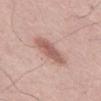site = the abdomen
imaging modality = total-body-photography crop, ~15 mm field of view
lesion diameter = ~4.5 mm (longest diameter)
subject = male, aged around 55